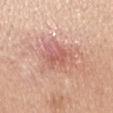• follow-up — no biopsy performed (imaged during a skin exam)
• patient — female, aged approximately 30
• diameter — about 3.5 mm
• tile lighting — white-light illumination
• imaging modality — ~15 mm tile from a whole-body skin photo
• location — the right upper arm